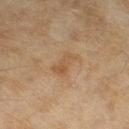Clinical impression:
No biopsy was performed on this lesion — it was imaged during a full skin examination and was not determined to be concerning.
Clinical summary:
The subject is a female roughly 60 years of age. Imaged with cross-polarized lighting. From the left lower leg. This image is a 15 mm lesion crop taken from a total-body photograph. The lesion-visualizer software estimated a classifier nevus-likeness of about 0/100 and a lesion-detection confidence of about 100/100.